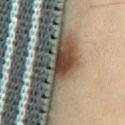This lesion was catalogued during total-body skin photography and was not selected for biopsy.
The patient is a male approximately 70 years of age.
From the front of the torso.
Cropped from a total-body skin-imaging series; the visible field is about 15 mm.